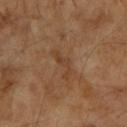biopsy status: total-body-photography surveillance lesion; no biopsy | image source: total-body-photography crop, ~15 mm field of view | patient: female, aged 68 to 72 | anatomic site: the arm | diameter: ~4 mm (longest diameter) | illumination: cross-polarized illumination.